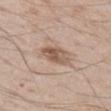<tbp_lesion>
<automated_metrics>
  <eccentricity>0.8</eccentricity>
  <border_irregularity_0_10>2.0</border_irregularity_0_10>
  <color_variation_0_10>5.0</color_variation_0_10>
  <peripheral_color_asymmetry>2.0</peripheral_color_asymmetry>
  <nevus_likeness_0_100>45</nevus_likeness_0_100>
</automated_metrics>
<patient>
  <sex>male</sex>
  <age_approx>65</age_approx>
</patient>
<site>left thigh</site>
<image>
  <source>total-body photography crop</source>
  <field_of_view_mm>15</field_of_view_mm>
</image>
</tbp_lesion>